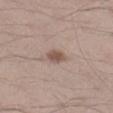{"biopsy_status": "not biopsied; imaged during a skin examination", "site": "right lower leg", "patient": {"sex": "male", "age_approx": 35}, "lesion_size": {"long_diameter_mm_approx": 2.5}, "image": {"source": "total-body photography crop", "field_of_view_mm": 15}, "automated_metrics": {"nevus_likeness_0_100": 85, "lesion_detection_confidence_0_100": 100}}